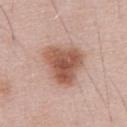Recorded during total-body skin imaging; not selected for excision or biopsy. The subject is a male roughly 55 years of age. A lesion tile, about 15 mm wide, cut from a 3D total-body photograph. The lesion is located on the front of the torso.